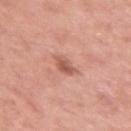Case summary:
* notes — total-body-photography surveillance lesion; no biopsy
* illumination — white-light illumination
* site — the arm
* acquisition — total-body-photography crop, ~15 mm field of view
* subject — female, in their 50s
* image-analysis metrics — an area of roughly 4 mm², an outline eccentricity of about 0.75 (0 = round, 1 = elongated), and a symmetry-axis asymmetry near 0.25; a nevus-likeness score of about 50/100 and lesion-presence confidence of about 100/100